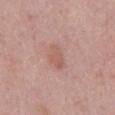biopsy_status: not biopsied; imaged during a skin examination
image:
  source: total-body photography crop
  field_of_view_mm: 15
site: abdomen
automated_metrics:
  area_mm2_approx: 4.5
  shape_asymmetry: 0.3
  vs_skin_contrast_norm: 5.0
lesion_size:
  long_diameter_mm_approx: 3.0
patient:
  sex: male
  age_approx: 50
lighting: white-light